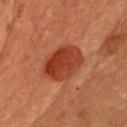Captured during whole-body skin photography for melanoma surveillance; the lesion was not biopsied. Approximately 5.5 mm at its widest. This is a cross-polarized tile. The subject is a male aged 73–77. A roughly 15 mm field-of-view crop from a total-body skin photograph. Automated tile analysis of the lesion measured an area of roughly 14 mm², a shape eccentricity near 0.75, and a symmetry-axis asymmetry near 0.15. It also reported a border-irregularity index near 1.5/10, a color-variation rating of about 4.5/10, and a peripheral color-asymmetry measure near 1.5. On the front of the torso.